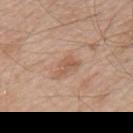<case>
<biopsy_status>not biopsied; imaged during a skin examination</biopsy_status>
<patient>
  <sex>male</sex>
  <age_approx>55</age_approx>
</patient>
<site>left upper arm</site>
<image>
  <source>total-body photography crop</source>
  <field_of_view_mm>15</field_of_view_mm>
</image>
</case>